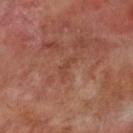patient = male, approximately 70 years of age | location = the left lower leg | size = about 2.5 mm | image source = 15 mm crop, total-body photography.